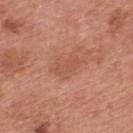Findings:
• automated lesion analysis — a footprint of about 4.5 mm², an eccentricity of roughly 0.85, and a symmetry-axis asymmetry near 0.3; a mean CIELAB color near L≈53 a*≈26 b*≈32 and a lesion–skin lightness drop of about 6; an automated nevus-likeness rating near 0 out of 100 and a lesion-detection confidence of about 100/100
• anatomic site — the upper back
• subject — male, roughly 70 years of age
• tile lighting — white-light illumination
• imaging modality — ~15 mm crop, total-body skin-cancer survey
• lesion diameter — ≈3 mm